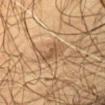biopsy status = imaged on a skin check; not biopsied
image = total-body-photography crop, ~15 mm field of view
TBP lesion metrics = an average lesion color of about L≈50 a*≈16 b*≈32 (CIELAB), about 8 CIELAB-L* units darker than the surrounding skin, and a normalized lesion–skin contrast near 6; border irregularity of about 3.5 on a 0–10 scale, a color-variation rating of about 3/10, and a peripheral color-asymmetry measure near 1; a lesion-detection confidence of about 60/100
lighting = cross-polarized illumination
lesion diameter = ≈3 mm
patient = male, roughly 55 years of age
body site = the arm